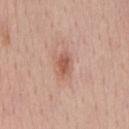Recorded during total-body skin imaging; not selected for excision or biopsy. Measured at roughly 3 mm in maximum diameter. A 15 mm close-up extracted from a 3D total-body photography capture. A male subject, approximately 55 years of age. On the chest.